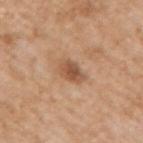Assessment: No biopsy was performed on this lesion — it was imaged during a full skin examination and was not determined to be concerning. Image and clinical context: About 3 mm across. A 15 mm close-up tile from a total-body photography series done for melanoma screening. The tile uses white-light illumination. The lesion is located on the right upper arm. A male patient in their mid- to late 60s.